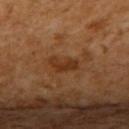<tbp_lesion>
<biopsy_status>not biopsied; imaged during a skin examination</biopsy_status>
<lighting>cross-polarized</lighting>
<image>
  <source>total-body photography crop</source>
  <field_of_view_mm>15</field_of_view_mm>
</image>
<patient>
  <sex>female</sex>
  <age_approx>65</age_approx>
</patient>
<lesion_size>
  <long_diameter_mm_approx>3.5</long_diameter_mm_approx>
</lesion_size>
<site>upper back</site>
</tbp_lesion>